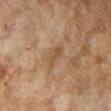notes: catalogued during a skin exam; not biopsied
lesion diameter: ~3 mm (longest diameter)
anatomic site: the left lower leg
automated lesion analysis: an average lesion color of about L≈45 a*≈15 b*≈30 (CIELAB), about 6 CIELAB-L* units darker than the surrounding skin, and a lesion-to-skin contrast of about 5.5 (normalized; higher = more distinct); an automated nevus-likeness rating near 0 out of 100 and a detector confidence of about 100 out of 100 that the crop contains a lesion
subject: female, aged approximately 60
lighting: cross-polarized illumination
image source: ~15 mm crop, total-body skin-cancer survey